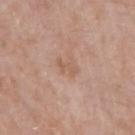This lesion was catalogued during total-body skin photography and was not selected for biopsy.
Measured at roughly 3 mm in maximum diameter.
This image is a 15 mm lesion crop taken from a total-body photograph.
The lesion is on the right upper arm.
Automated tile analysis of the lesion measured an automated nevus-likeness rating near 0 out of 100 and lesion-presence confidence of about 100/100.
A male patient about 65 years old.